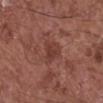Notes:
– workup — imaged on a skin check; not biopsied
– patient — male, aged 63–67
– illumination — white-light illumination
– site — the right lower leg
– imaging modality — total-body-photography crop, ~15 mm field of view
– lesion diameter — ~3 mm (longest diameter)